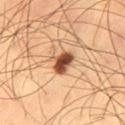Part of a total-body skin-imaging series; this lesion was reviewed on a skin check and was not flagged for biopsy. Cropped from a whole-body photographic skin survey; the tile spans about 15 mm. The tile uses cross-polarized illumination. Approximately 3.5 mm at its widest. The lesion is on the left thigh. The subject is a male aged 58–62.